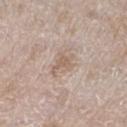Case summary:
- biopsy status · total-body-photography surveillance lesion; no biopsy
- body site · the left lower leg
- subject · female, about 75 years old
- acquisition · ~15 mm crop, total-body skin-cancer survey
- automated lesion analysis · a lesion area of about 6 mm², an outline eccentricity of about 0.8 (0 = round, 1 = elongated), and two-axis asymmetry of about 0.35; a lesion color around L≈61 a*≈14 b*≈25 in CIELAB, about 8 CIELAB-L* units darker than the surrounding skin, and a lesion-to-skin contrast of about 5.5 (normalized; higher = more distinct); a border-irregularity rating of about 4/10, a color-variation rating of about 2/10, and peripheral color asymmetry of about 0.5